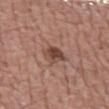biopsy status: total-body-photography surveillance lesion; no biopsy | anatomic site: the left upper arm | image: 15 mm crop, total-body photography | subject: male, in their 70s.